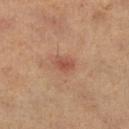{
  "lesion_size": {
    "long_diameter_mm_approx": 2.5
  },
  "patient": {
    "sex": "female",
    "age_approx": 40
  },
  "site": "right lower leg",
  "image": {
    "source": "total-body photography crop",
    "field_of_view_mm": 15
  },
  "lighting": "cross-polarized"
}